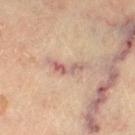Clinical impression: This lesion was catalogued during total-body skin photography and was not selected for biopsy. Context: A 15 mm crop from a total-body photograph taken for skin-cancer surveillance. On the left lower leg. The lesion-visualizer software estimated an area of roughly 5.5 mm² and an outline eccentricity of about 0.95 (0 = round, 1 = elongated). The analysis additionally found a mean CIELAB color near L≈52 a*≈17 b*≈22, about 8 CIELAB-L* units darker than the surrounding skin, and a normalized lesion–skin contrast near 7. It also reported a border-irregularity rating of about 7/10, a within-lesion color-variation index near 4/10, and a peripheral color-asymmetry measure near 1. And it measured a nevus-likeness score of about 0/100. Captured under cross-polarized illumination. A female patient, aged 78 to 82.